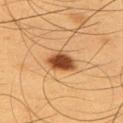body site = the back
lighting = cross-polarized illumination
subject = male, aged 53–57
automated lesion analysis = a lesion area of about 7.5 mm² and two-axis asymmetry of about 0.2; a lesion color around L≈48 a*≈25 b*≈39 in CIELAB and roughly 18 lightness units darker than nearby skin
lesion size = ≈3.5 mm
imaging modality = ~15 mm crop, total-body skin-cancer survey